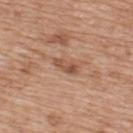{"biopsy_status": "not biopsied; imaged during a skin examination", "image": {"source": "total-body photography crop", "field_of_view_mm": 15}, "site": "upper back", "patient": {"sex": "male", "age_approx": 50}}